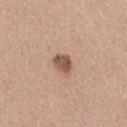No biopsy was performed on this lesion — it was imaged during a full skin examination and was not determined to be concerning. The lesion is located on the right thigh. Captured under white-light illumination. A close-up tile cropped from a whole-body skin photograph, about 15 mm across. The recorded lesion diameter is about 2.5 mm. A female patient in their mid-40s.